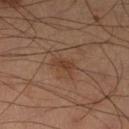– biopsy status — no biopsy performed (imaged during a skin exam)
– subject — male, aged 58 to 62
– size — ~2.5 mm (longest diameter)
– imaging modality — ~15 mm crop, total-body skin-cancer survey
– site — the left lower leg